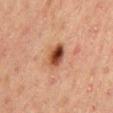  biopsy_status: not biopsied; imaged during a skin examination
  lesion_size:
    long_diameter_mm_approx: 3.5
  patient:
    sex: male
    age_approx: 50
  lighting: cross-polarized
  image:
    source: total-body photography crop
    field_of_view_mm: 15
  site: back